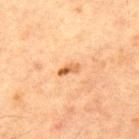Cropped from a total-body skin-imaging series; the visible field is about 15 mm. The lesion is on the chest. A male subject roughly 65 years of age.